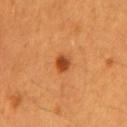follow-up: imaged on a skin check; not biopsied
acquisition: total-body-photography crop, ~15 mm field of view
lighting: cross-polarized illumination
subject: male, aged around 60
lesion size: about 2.5 mm
body site: the mid back
TBP lesion metrics: a lesion area of about 4 mm² and an outline eccentricity of about 0.65 (0 = round, 1 = elongated); a lesion color around L≈44 a*≈29 b*≈40 in CIELAB, roughly 12 lightness units darker than nearby skin, and a normalized lesion–skin contrast near 9